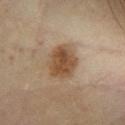No biopsy was performed on this lesion — it was imaged during a full skin examination and was not determined to be concerning. On the left lower leg. A 15 mm crop from a total-body photograph taken for skin-cancer surveillance. The patient is a male about 60 years old. About 4.5 mm across. The tile uses cross-polarized illumination.